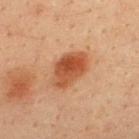biopsy status: total-body-photography surveillance lesion; no biopsy | anatomic site: the upper back | lighting: cross-polarized illumination | subject: male, aged 33 to 37 | imaging modality: 15 mm crop, total-body photography | size: ~5 mm (longest diameter).